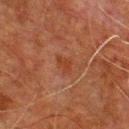- follow-up · total-body-photography surveillance lesion; no biopsy
- image · total-body-photography crop, ~15 mm field of view
- illumination · cross-polarized illumination
- body site · the chest
- subject · male, roughly 80 years of age